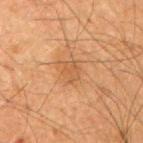Clinical impression:
Imaged during a routine full-body skin examination; the lesion was not biopsied and no histopathology is available.
Acquisition and patient details:
A male patient aged 63 to 67. The lesion is on the right upper arm. A 15 mm close-up tile from a total-body photography series done for melanoma screening.